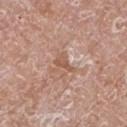patient: male, roughly 60 years of age | image-analysis metrics: an average lesion color of about L≈55 a*≈21 b*≈29 (CIELAB) and a lesion-to-skin contrast of about 6.5 (normalized; higher = more distinct); a border-irregularity rating of about 5/10 and a peripheral color-asymmetry measure near 0.5; an automated nevus-likeness rating near 0 out of 100 and a detector confidence of about 85 out of 100 that the crop contains a lesion | lesion size: ≈3 mm | site: the leg | image source: total-body-photography crop, ~15 mm field of view.